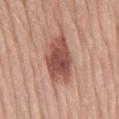lighting: white-light
TBP lesion metrics: a lesion area of about 17 mm² and a shape eccentricity near 0.8; a mean CIELAB color near L≈51 a*≈24 b*≈27, about 14 CIELAB-L* units darker than the surrounding skin, and a normalized lesion–skin contrast near 9.5; a color-variation rating of about 4.5/10
image source: total-body-photography crop, ~15 mm field of view
location: the mid back
subject: male, aged approximately 65